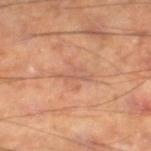Impression:
Recorded during total-body skin imaging; not selected for excision or biopsy.
Acquisition and patient details:
Longest diameter approximately 3.5 mm. Automated image analysis of the tile measured border irregularity of about 10 on a 0–10 scale, internal color variation of about 0 on a 0–10 scale, and a peripheral color-asymmetry measure near 0. Located on the right lower leg. A region of skin cropped from a whole-body photographic capture, roughly 15 mm wide. A male subject, approximately 55 years of age. This is a cross-polarized tile.